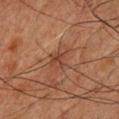{"biopsy_status": "not biopsied; imaged during a skin examination", "patient": {"sex": "male", "age_approx": 60}, "site": "chest", "image": {"source": "total-body photography crop", "field_of_view_mm": 15}}